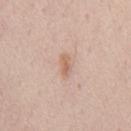Clinical impression:
Part of a total-body skin-imaging series; this lesion was reviewed on a skin check and was not flagged for biopsy.
Context:
From the chest. The tile uses white-light illumination. A 15 mm close-up tile from a total-body photography series done for melanoma screening. Approximately 2.5 mm at its widest. A male patient, aged around 45.